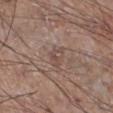Captured during whole-body skin photography for melanoma surveillance; the lesion was not biopsied. A male subject roughly 65 years of age. On the right lower leg. About 3 mm across. Captured under white-light illumination. A region of skin cropped from a whole-body photographic capture, roughly 15 mm wide.